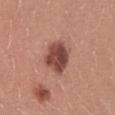biopsy_status: not biopsied; imaged during a skin examination
patient:
  sex: female
  age_approx: 25
site: abdomen
automated_metrics:
  area_mm2_approx: 11.0
  eccentricity: 0.5
  shape_asymmetry: 0.2
  border_irregularity_0_10: 2.0
  color_variation_0_10: 5.0
  peripheral_color_asymmetry: 1.5
image:
  source: total-body photography crop
  field_of_view_mm: 15
lesion_size:
  long_diameter_mm_approx: 4.0